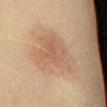workup: catalogued during a skin exam; not biopsied
subject: female, roughly 50 years of age
imaging modality: total-body-photography crop, ~15 mm field of view
location: the right lower leg